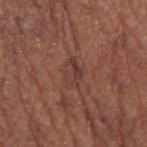biopsy status: total-body-photography surveillance lesion; no biopsy
lesion size: about 3.5 mm
subject: female, approximately 75 years of age
lighting: white-light illumination
acquisition: 15 mm crop, total-body photography
automated lesion analysis: border irregularity of about 3.5 on a 0–10 scale, a color-variation rating of about 4/10, and a peripheral color-asymmetry measure near 1.5
site: the arm